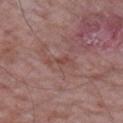Part of a total-body skin-imaging series; this lesion was reviewed on a skin check and was not flagged for biopsy. A close-up tile cropped from a whole-body skin photograph, about 15 mm across. A male subject, aged 63–67. The lesion is on the right upper arm.